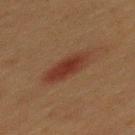No biopsy was performed on this lesion — it was imaged during a full skin examination and was not determined to be concerning. A male patient approximately 40 years of age. On the mid back. A 15 mm crop from a total-body photograph taken for skin-cancer surveillance.